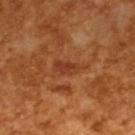Q: Is there a histopathology result?
A: total-body-photography surveillance lesion; no biopsy
Q: Who is the patient?
A: male, about 65 years old
Q: Automated lesion metrics?
A: a mean CIELAB color near L≈38 a*≈27 b*≈35 and a normalized border contrast of about 6; a detector confidence of about 95 out of 100 that the crop contains a lesion
Q: What is the imaging modality?
A: ~15 mm tile from a whole-body skin photo
Q: What lighting was used for the tile?
A: cross-polarized illumination